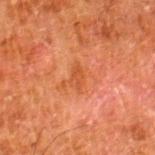Assessment: Part of a total-body skin-imaging series; this lesion was reviewed on a skin check and was not flagged for biopsy. Background: A male subject roughly 80 years of age. Automated tile analysis of the lesion measured a lesion color around L≈42 a*≈27 b*≈35 in CIELAB, roughly 6 lightness units darker than nearby skin, and a lesion-to-skin contrast of about 5.5 (normalized; higher = more distinct). The software also gave a border-irregularity index near 4/10, internal color variation of about 1.5 on a 0–10 scale, and a peripheral color-asymmetry measure near 0.5. The analysis additionally found a classifier nevus-likeness of about 0/100 and a lesion-detection confidence of about 100/100. Imaged with cross-polarized lighting. The lesion's longest dimension is about 3 mm. The lesion is on the right lower leg. A 15 mm close-up tile from a total-body photography series done for melanoma screening.